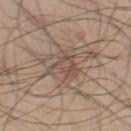Assessment: No biopsy was performed on this lesion — it was imaged during a full skin examination and was not determined to be concerning.